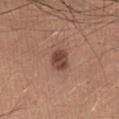Q: Is there a histopathology result?
A: no biopsy performed (imaged during a skin exam)
Q: How was the tile lit?
A: white-light illumination
Q: What is the anatomic site?
A: the front of the torso
Q: What is the lesion's diameter?
A: ~2.5 mm (longest diameter)
Q: What are the patient's age and sex?
A: male, in their mid- to late 20s
Q: What kind of image is this?
A: ~15 mm tile from a whole-body skin photo